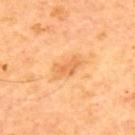Case summary:
- biopsy status · no biopsy performed (imaged during a skin exam)
- body site · the upper back
- illumination · cross-polarized illumination
- patient · male, approximately 65 years of age
- size · about 3.5 mm
- image source · ~15 mm crop, total-body skin-cancer survey